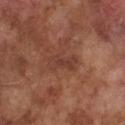workup: catalogued during a skin exam; not biopsied | body site: the chest | lighting: white-light illumination | image: total-body-photography crop, ~15 mm field of view | subject: male, in their mid-70s.